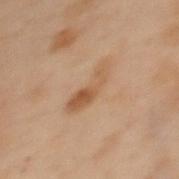Assessment: Part of a total-body skin-imaging series; this lesion was reviewed on a skin check and was not flagged for biopsy. Background: Imaged with cross-polarized lighting. A female subject, in their mid- to late 50s. The total-body-photography lesion software estimated a border-irregularity rating of about 4/10, internal color variation of about 6 on a 0–10 scale, and radial color variation of about 2. And it measured a classifier nevus-likeness of about 0/100 and lesion-presence confidence of about 100/100. A close-up tile cropped from a whole-body skin photograph, about 15 mm across. The lesion is on the upper back.